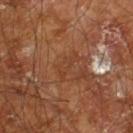Imaged during a routine full-body skin examination; the lesion was not biopsied and no histopathology is available. The recorded lesion diameter is about 3.5 mm. A male patient in their 60s. Captured under cross-polarized illumination. The lesion is located on the right leg. Automated tile analysis of the lesion measured a lesion area of about 4 mm², an eccentricity of roughly 0.9, and a shape-asymmetry score of about 0.45 (0 = symmetric). It also reported an average lesion color of about L≈39 a*≈22 b*≈32 (CIELAB), a lesion–skin lightness drop of about 5, and a lesion-to-skin contrast of about 4.5 (normalized; higher = more distinct). The analysis additionally found a border-irregularity index near 6/10, internal color variation of about 0 on a 0–10 scale, and peripheral color asymmetry of about 0. The analysis additionally found a detector confidence of about 90 out of 100 that the crop contains a lesion. A 15 mm close-up extracted from a 3D total-body photography capture.